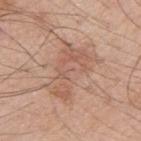anatomic site = the right upper arm | patient = male, aged around 60 | TBP lesion metrics = a lesion color around L≈58 a*≈20 b*≈28 in CIELAB and roughly 8 lightness units darker than nearby skin; a border-irregularity rating of about 6.5/10 and peripheral color asymmetry of about 1 | lesion diameter = ≈6.5 mm | image source = 15 mm crop, total-body photography.